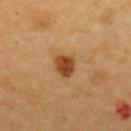Assessment: This lesion was catalogued during total-body skin photography and was not selected for biopsy. Acquisition and patient details: Cropped from a whole-body photographic skin survey; the tile spans about 15 mm. The tile uses cross-polarized illumination. The patient is a female about 60 years old. From the back. The lesion-visualizer software estimated an area of roughly 5.5 mm², an eccentricity of roughly 0.55, and a shape-asymmetry score of about 0.25 (0 = symmetric). The software also gave a lesion–skin lightness drop of about 14 and a lesion-to-skin contrast of about 10 (normalized; higher = more distinct). The recorded lesion diameter is about 3 mm.